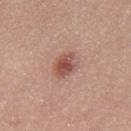| key | value |
|---|---|
| follow-up | imaged on a skin check; not biopsied |
| lighting | white-light |
| lesion size | ≈3 mm |
| location | the back |
| image | ~15 mm crop, total-body skin-cancer survey |
| subject | male, in their mid-20s |
| TBP lesion metrics | an area of roughly 6 mm² and an outline eccentricity of about 0.7 (0 = round, 1 = elongated); a mean CIELAB color near L≈51 a*≈24 b*≈26, roughly 12 lightness units darker than nearby skin, and a lesion-to-skin contrast of about 8.5 (normalized; higher = more distinct); border irregularity of about 2 on a 0–10 scale and radial color variation of about 1; an automated nevus-likeness rating near 90 out of 100 and lesion-presence confidence of about 100/100 |